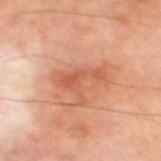This is a cross-polarized tile.
A male subject in their 70s.
The recorded lesion diameter is about 4.5 mm.
Located on the left thigh.
A 15 mm close-up extracted from a 3D total-body photography capture.
The lesion-visualizer software estimated a footprint of about 8 mm² and two-axis asymmetry of about 0.55.